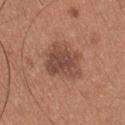workup: imaged on a skin check; not biopsied | location: the left upper arm | subject: male, aged 33–37 | imaging modality: ~15 mm tile from a whole-body skin photo.